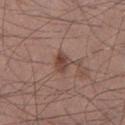biopsy status: imaged on a skin check; not biopsied.